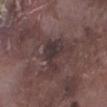Clinical impression: The lesion was tiled from a total-body skin photograph and was not biopsied. Acquisition and patient details: A region of skin cropped from a whole-body photographic capture, roughly 15 mm wide. From the leg. A male subject in their mid-70s.